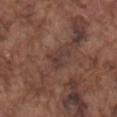biopsy status = total-body-photography surveillance lesion; no biopsy | subject = male, about 75 years old | size = ~3 mm (longest diameter) | acquisition = total-body-photography crop, ~15 mm field of view | body site = the mid back | tile lighting = white-light illumination.